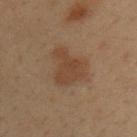On the left upper arm.
A male patient, in their mid- to late 30s.
A region of skin cropped from a whole-body photographic capture, roughly 15 mm wide.
Imaged with cross-polarized lighting.
Approximately 4 mm at its widest.